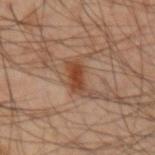notes: imaged on a skin check; not biopsied
image source: ~15 mm crop, total-body skin-cancer survey
patient: male, roughly 50 years of age
lesion diameter: about 3.5 mm
body site: the left arm
tile lighting: cross-polarized